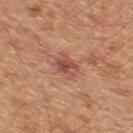Assessment: The lesion was photographed on a routine skin check and not biopsied; there is no pathology result. Background: A region of skin cropped from a whole-body photographic capture, roughly 15 mm wide. The lesion's longest dimension is about 3 mm. The lesion is located on the back. This is a white-light tile. A male subject aged approximately 45.